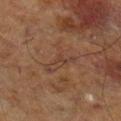Clinical impression: Recorded during total-body skin imaging; not selected for excision or biopsy. Image and clinical context: A male patient aged 68–72. An algorithmic analysis of the crop reported a border-irregularity index near 7.5/10. This is a cross-polarized tile. On the right lower leg. A 15 mm close-up tile from a total-body photography series done for melanoma screening.